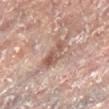Recorded during total-body skin imaging; not selected for excision or biopsy. The patient is a female aged approximately 80. On the right leg. This image is a 15 mm lesion crop taken from a total-body photograph.